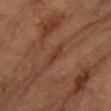Captured during whole-body skin photography for melanoma surveillance; the lesion was not biopsied.